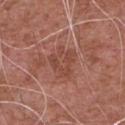Clinical impression: Captured during whole-body skin photography for melanoma surveillance; the lesion was not biopsied.